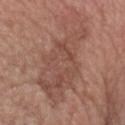Findings:
* notes: catalogued during a skin exam; not biopsied
* lesion diameter: about 11 mm
* subject: male, aged 63 to 67
* site: the head or neck
* tile lighting: white-light illumination
* image source: ~15 mm crop, total-body skin-cancer survey